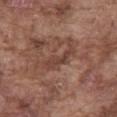notes: no biopsy performed (imaged during a skin exam); subject: male, aged approximately 75; image source: ~15 mm tile from a whole-body skin photo; lighting: white-light; anatomic site: the abdomen.